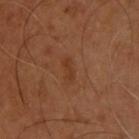Recorded during total-body skin imaging; not selected for excision or biopsy. Automated image analysis of the tile measured a footprint of about 2.5 mm², an eccentricity of roughly 0.95, and a shape-asymmetry score of about 0.2 (0 = symmetric). The lesion is located on the upper back. A male patient in their mid- to late 50s. A 15 mm close-up extracted from a 3D total-body photography capture. About 2.5 mm across.